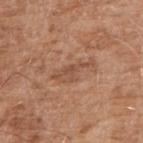Notes:
– workup · total-body-photography surveillance lesion; no biopsy
– location · the right upper arm
– image source · 15 mm crop, total-body photography
– patient · male, in their 60s